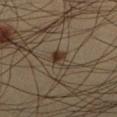Case summary:
- imaging modality — ~15 mm crop, total-body skin-cancer survey
- subject — male, in their mid-30s
- lighting — cross-polarized illumination
- anatomic site — the right lower leg
- TBP lesion metrics — border irregularity of about 3.5 on a 0–10 scale and internal color variation of about 4 on a 0–10 scale